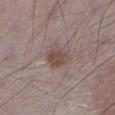Part of a total-body skin-imaging series; this lesion was reviewed on a skin check and was not flagged for biopsy.
From the leg.
A male patient aged 68–72.
The lesion's longest dimension is about 3 mm.
The tile uses white-light illumination.
The total-body-photography lesion software estimated a mean CIELAB color near L≈47 a*≈16 b*≈21, roughly 9 lightness units darker than nearby skin, and a normalized border contrast of about 7.5. The software also gave border irregularity of about 1.5 on a 0–10 scale, a color-variation rating of about 2.5/10, and peripheral color asymmetry of about 1. The software also gave lesion-presence confidence of about 100/100.
A region of skin cropped from a whole-body photographic capture, roughly 15 mm wide.